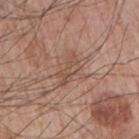About 4 mm across.
Cropped from a total-body skin-imaging series; the visible field is about 15 mm.
From the right upper arm.
Imaged with white-light lighting.
Automated tile analysis of the lesion measured a lesion area of about 5.5 mm² and a shape-asymmetry score of about 0.5 (0 = symmetric). And it measured an average lesion color of about L≈51 a*≈18 b*≈27 (CIELAB), a lesion–skin lightness drop of about 7, and a lesion-to-skin contrast of about 5 (normalized; higher = more distinct). The analysis additionally found a lesion-detection confidence of about 95/100.
A male patient, aged 63–67.